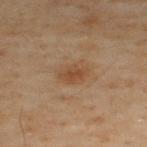follow-up: imaged on a skin check; not biopsied | site: the upper back | lighting: cross-polarized illumination | lesion diameter: ≈3 mm | subject: male, aged 48 to 52 | automated lesion analysis: a border-irregularity rating of about 1.5/10, a color-variation rating of about 2.5/10, and radial color variation of about 1; a nevus-likeness score of about 55/100 and a lesion-detection confidence of about 100/100 | acquisition: 15 mm crop, total-body photography.